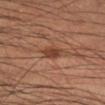A 15 mm close-up tile from a total-body photography series done for melanoma screening. The lesion is on the right lower leg. A male subject, roughly 45 years of age. The recorded lesion diameter is about 3 mm.